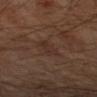- biopsy status: no biopsy performed (imaged during a skin exam)
- location: the left upper arm
- subject: male, aged 68–72
- lesion size: ≈3 mm
- illumination: cross-polarized
- image source: ~15 mm crop, total-body skin-cancer survey
- automated lesion analysis: roughly 4 lightness units darker than nearby skin and a normalized border contrast of about 5